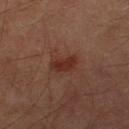workup = no biopsy performed (imaged during a skin exam)
image source = 15 mm crop, total-body photography
anatomic site = the right thigh
illumination = cross-polarized illumination
automated lesion analysis = an average lesion color of about L≈27 a*≈22 b*≈23 (CIELAB) and a lesion–skin lightness drop of about 8; a nevus-likeness score of about 55/100
lesion size = ≈3 mm
subject = male, aged approximately 60